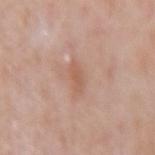Imaged during a routine full-body skin examination; the lesion was not biopsied and no histopathology is available. An algorithmic analysis of the crop reported an outline eccentricity of about 0.85 (0 = round, 1 = elongated) and a shape-asymmetry score of about 0.25 (0 = symmetric). The analysis additionally found border irregularity of about 2.5 on a 0–10 scale, internal color variation of about 1 on a 0–10 scale, and a peripheral color-asymmetry measure near 0.5. It also reported an automated nevus-likeness rating near 0 out of 100 and a lesion-detection confidence of about 100/100. On the right forearm. The patient is a female aged approximately 65. About 2.5 mm across. A roughly 15 mm field-of-view crop from a total-body skin photograph. Imaged with white-light lighting.